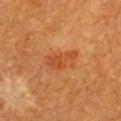Case summary:
- follow-up · no biopsy performed (imaged during a skin exam)
- subject · female, roughly 55 years of age
- acquisition · ~15 mm crop, total-body skin-cancer survey
- anatomic site · the chest
- lesion diameter · ≈4 mm
- illumination · cross-polarized